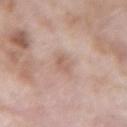Captured during whole-body skin photography for melanoma surveillance; the lesion was not biopsied. The lesion is located on the left forearm. Automated tile analysis of the lesion measured a footprint of about 4 mm², a shape eccentricity near 0.7, and a symmetry-axis asymmetry near 0.25. It also reported a mean CIELAB color near L≈61 a*≈18 b*≈26 and roughly 7 lightness units darker than nearby skin. The analysis additionally found a nevus-likeness score of about 5/100. Longest diameter approximately 2.5 mm. A lesion tile, about 15 mm wide, cut from a 3D total-body photograph. Imaged with white-light lighting. A male patient, approximately 60 years of age.